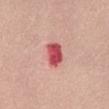| feature | finding |
|---|---|
| follow-up | total-body-photography surveillance lesion; no biopsy |
| acquisition | 15 mm crop, total-body photography |
| tile lighting | white-light |
| patient | female, roughly 55 years of age |
| site | the front of the torso |
| lesion diameter | ~3 mm (longest diameter) |